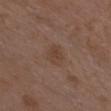Clinical impression: The lesion was photographed on a routine skin check and not biopsied; there is no pathology result. Image and clinical context: This image is a 15 mm lesion crop taken from a total-body photograph. A female subject, aged 33–37. Approximately 3 mm at its widest. This is a white-light tile. Located on the chest. The total-body-photography lesion software estimated a lesion area of about 5.5 mm², an eccentricity of roughly 0.6, and a shape-asymmetry score of about 0.15 (0 = symmetric). It also reported border irregularity of about 1.5 on a 0–10 scale and a peripheral color-asymmetry measure near 1. The analysis additionally found an automated nevus-likeness rating near 10 out of 100.